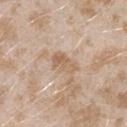  patient:
    sex: female
    age_approx: 25
  site: left upper arm
  automated_metrics:
    eccentricity: 0.8
    shape_asymmetry: 0.25
    cielab_L: 61
    cielab_a: 16
    cielab_b: 31
    vs_skin_darker_L: 8.0
  image:
    source: total-body photography crop
    field_of_view_mm: 15